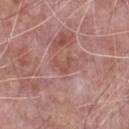biopsy status — no biopsy performed (imaged during a skin exam).